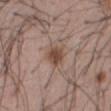Impression: Part of a total-body skin-imaging series; this lesion was reviewed on a skin check and was not flagged for biopsy. Context: Longest diameter approximately 2.5 mm. Captured under white-light illumination. The patient is a male aged approximately 55. The lesion is located on the abdomen. The lesion-visualizer software estimated a footprint of about 6 mm², an outline eccentricity of about 0.4 (0 = round, 1 = elongated), and two-axis asymmetry of about 0.2. It also reported a mean CIELAB color near L≈47 a*≈18 b*≈25, roughly 10 lightness units darker than nearby skin, and a normalized border contrast of about 8.5. The software also gave a border-irregularity rating of about 2/10, a within-lesion color-variation index near 2.5/10, and peripheral color asymmetry of about 1. And it measured a classifier nevus-likeness of about 90/100 and lesion-presence confidence of about 100/100. A lesion tile, about 15 mm wide, cut from a 3D total-body photograph.